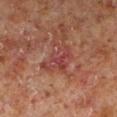Imaged during a routine full-body skin examination; the lesion was not biopsied and no histopathology is available. On the leg. This image is a 15 mm lesion crop taken from a total-body photograph. A male subject about 60 years old.